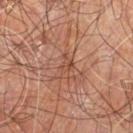workup = catalogued during a skin exam; not biopsied
patient = male, aged around 60
tile lighting = cross-polarized illumination
anatomic site = the right leg
automated lesion analysis = a lesion area of about 3.5 mm², an eccentricity of roughly 0.85, and a symmetry-axis asymmetry near 0.55; a nevus-likeness score of about 0/100 and lesion-presence confidence of about 50/100
image source = total-body-photography crop, ~15 mm field of view
lesion diameter = about 3 mm